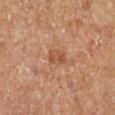This lesion was catalogued during total-body skin photography and was not selected for biopsy. Located on the left lower leg. This image is a 15 mm lesion crop taken from a total-body photograph. The patient is female. The tile uses cross-polarized illumination.